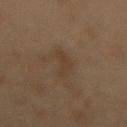notes: no biopsy performed (imaged during a skin exam); lighting: cross-polarized; location: the mid back; imaging modality: ~15 mm tile from a whole-body skin photo; patient: female, in their mid- to late 50s; size: about 4 mm.